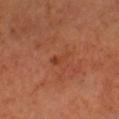Q: Was a biopsy performed?
A: no biopsy performed (imaged during a skin exam)
Q: What is the imaging modality?
A: ~15 mm tile from a whole-body skin photo
Q: What did automated image analysis measure?
A: a footprint of about 2.5 mm² and an outline eccentricity of about 0.85 (0 = round, 1 = elongated); an average lesion color of about L≈42 a*≈28 b*≈34 (CIELAB), a lesion–skin lightness drop of about 5, and a normalized border contrast of about 4.5; a nevus-likeness score of about 0/100 and a detector confidence of about 100 out of 100 that the crop contains a lesion
Q: What lighting was used for the tile?
A: cross-polarized illumination
Q: Lesion size?
A: ~2.5 mm (longest diameter)
Q: Where on the body is the lesion?
A: the head or neck
Q: Who is the patient?
A: male, about 40 years old